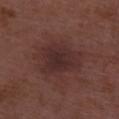Captured during whole-body skin photography for melanoma surveillance; the lesion was not biopsied. A roughly 15 mm field-of-view crop from a total-body skin photograph. A male patient, roughly 50 years of age. Captured under white-light illumination.